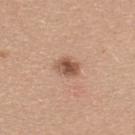Imaged during a routine full-body skin examination; the lesion was not biopsied and no histopathology is available. The lesion is located on the upper back. Longest diameter approximately 3 mm. A close-up tile cropped from a whole-body skin photograph, about 15 mm across. A female subject, aged approximately 25.